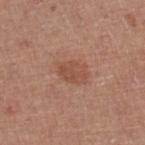Clinical impression:
Imaged during a routine full-body skin examination; the lesion was not biopsied and no histopathology is available.
Acquisition and patient details:
The total-body-photography lesion software estimated a footprint of about 7.5 mm². And it measured an average lesion color of about L≈50 a*≈23 b*≈29 (CIELAB), about 7 CIELAB-L* units darker than the surrounding skin, and a lesion-to-skin contrast of about 5.5 (normalized; higher = more distinct). The analysis additionally found a border-irregularity rating of about 2/10, a color-variation rating of about 2.5/10, and a peripheral color-asymmetry measure near 1. A female subject, about 50 years old. About 3.5 mm across. Captured under white-light illumination. From the right lower leg. Cropped from a total-body skin-imaging series; the visible field is about 15 mm.